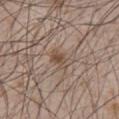{
  "biopsy_status": "not biopsied; imaged during a skin examination",
  "lesion_size": {
    "long_diameter_mm_approx": 3.0
  },
  "automated_metrics": {
    "area_mm2_approx": 4.0,
    "shape_asymmetry": 0.3,
    "vs_skin_darker_L": 8.0,
    "vs_skin_contrast_norm": 7.0,
    "nevus_likeness_0_100": 45,
    "lesion_detection_confidence_0_100": 100
  },
  "patient": {
    "sex": "male",
    "age_approx": 65
  },
  "site": "front of the torso",
  "lighting": "white-light",
  "image": {
    "source": "total-body photography crop",
    "field_of_view_mm": 15
  }
}